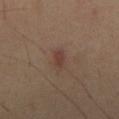• biopsy status — total-body-photography surveillance lesion; no biopsy
• body site — the chest
• illumination — cross-polarized
• acquisition — ~15 mm crop, total-body skin-cancer survey
• size — about 3 mm
• subject — male, approximately 45 years of age